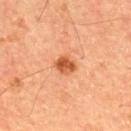Q: Was a biopsy performed?
A: imaged on a skin check; not biopsied
Q: What are the patient's age and sex?
A: male, aged 58–62
Q: Automated lesion metrics?
A: an outline eccentricity of about 0.6 (0 = round, 1 = elongated); an average lesion color of about L≈50 a*≈27 b*≈38 (CIELAB), about 13 CIELAB-L* units darker than the surrounding skin, and a normalized border contrast of about 9; a border-irregularity index near 1/10, a within-lesion color-variation index near 3.5/10, and peripheral color asymmetry of about 1
Q: What is the lesion's diameter?
A: ≈2.5 mm
Q: How was the tile lit?
A: cross-polarized illumination
Q: What kind of image is this?
A: total-body-photography crop, ~15 mm field of view